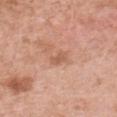Assessment:
Captured during whole-body skin photography for melanoma surveillance; the lesion was not biopsied.
Acquisition and patient details:
Imaged with white-light lighting. A roughly 15 mm field-of-view crop from a total-body skin photograph. A female patient, aged around 50. On the chest.